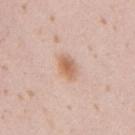Assessment:
Part of a total-body skin-imaging series; this lesion was reviewed on a skin check and was not flagged for biopsy.